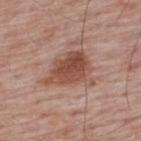Q: Was a biopsy performed?
A: no biopsy performed (imaged during a skin exam)
Q: Where on the body is the lesion?
A: the upper back
Q: How was the tile lit?
A: white-light
Q: What did automated image analysis measure?
A: a footprint of about 16 mm², a shape eccentricity near 0.7, and a symmetry-axis asymmetry near 0.4
Q: What is the imaging modality?
A: 15 mm crop, total-body photography
Q: What are the patient's age and sex?
A: male, aged 63 to 67